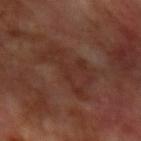The lesion was photographed on a routine skin check and not biopsied; there is no pathology result. A male patient, in their 70s. The lesion is on the left upper arm. A close-up tile cropped from a whole-body skin photograph, about 15 mm across.